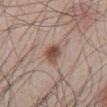Impression: This lesion was catalogued during total-body skin photography and was not selected for biopsy. Acquisition and patient details: The tile uses white-light illumination. Measured at roughly 2.5 mm in maximum diameter. A 15 mm close-up extracted from a 3D total-body photography capture. The lesion is on the front of the torso. A male subject aged 48–52.